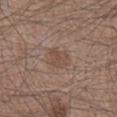workup: imaged on a skin check; not biopsied | tile lighting: white-light illumination | site: the right lower leg | lesion size: ≈2.5 mm | image source: ~15 mm tile from a whole-body skin photo | subject: male, aged around 45 | image-analysis metrics: a lesion area of about 5 mm² and a shape eccentricity near 0.6; an automated nevus-likeness rating near 15 out of 100.